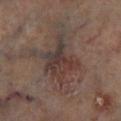This lesion was catalogued during total-body skin photography and was not selected for biopsy. On the right lower leg. A close-up tile cropped from a whole-body skin photograph, about 15 mm across. The tile uses cross-polarized illumination. About 6 mm across. A male patient, approximately 65 years of age. Automated image analysis of the tile measured an average lesion color of about L≈34 a*≈13 b*≈18 (CIELAB). The software also gave a border-irregularity rating of about 6/10, internal color variation of about 7.5 on a 0–10 scale, and radial color variation of about 2.5.